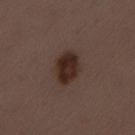Assessment: Captured during whole-body skin photography for melanoma surveillance; the lesion was not biopsied. Context: An algorithmic analysis of the crop reported a lesion area of about 8.5 mm², a shape eccentricity near 0.7, and two-axis asymmetry of about 0.15. It also reported an average lesion color of about L≈26 a*≈16 b*≈20 (CIELAB), roughly 11 lightness units darker than nearby skin, and a normalized border contrast of about 12. The tile uses white-light illumination. A female subject aged 48 to 52. On the lower back. Cropped from a whole-body photographic skin survey; the tile spans about 15 mm.